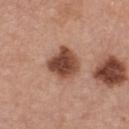Background: The lesion-visualizer software estimated an area of roughly 12 mm² and an outline eccentricity of about 0.3 (0 = round, 1 = elongated). The analysis additionally found an average lesion color of about L≈47 a*≈22 b*≈29 (CIELAB) and about 16 CIELAB-L* units darker than the surrounding skin. The software also gave a border-irregularity rating of about 1.5/10, internal color variation of about 5 on a 0–10 scale, and peripheral color asymmetry of about 1.5. The tile uses white-light illumination. The patient is a female aged around 45. Cropped from a whole-body photographic skin survey; the tile spans about 15 mm. The lesion is on the chest.